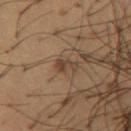Clinical impression: Recorded during total-body skin imaging; not selected for excision or biopsy. Acquisition and patient details: The lesion is located on the chest. A male subject about 50 years old. Imaged with cross-polarized lighting. A 15 mm close-up extracted from a 3D total-body photography capture. An algorithmic analysis of the crop reported a lesion area of about 3 mm², an eccentricity of roughly 0.85, and a symmetry-axis asymmetry near 0.4. And it measured about 7 CIELAB-L* units darker than the surrounding skin and a normalized lesion–skin contrast near 7.